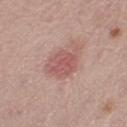This lesion was catalogued during total-body skin photography and was not selected for biopsy. A female patient about 65 years old. The recorded lesion diameter is about 5 mm. Captured under white-light illumination. A close-up tile cropped from a whole-body skin photograph, about 15 mm across. The lesion is located on the right thigh.